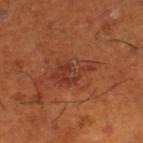Findings:
* notes — imaged on a skin check; not biopsied
* lighting — cross-polarized
* subject — male, aged around 65
* acquisition — total-body-photography crop, ~15 mm field of view
* location — the right lower leg
* lesion size — about 5 mm